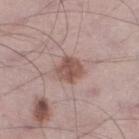Case summary:
• biopsy status · imaged on a skin check; not biopsied
• automated metrics · an average lesion color of about L≈52 a*≈19 b*≈23 (CIELAB), about 11 CIELAB-L* units darker than the surrounding skin, and a normalized border contrast of about 8; a border-irregularity index near 2.5/10, a color-variation rating of about 3/10, and radial color variation of about 1
• location · the left lower leg
• diameter · about 3.5 mm
• imaging modality · ~15 mm tile from a whole-body skin photo
• subject · male, about 70 years old
• lighting · white-light illumination